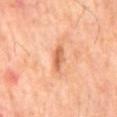No biopsy was performed on this lesion — it was imaged during a full skin examination and was not determined to be concerning.
A male subject aged around 65.
From the mid back.
Cropped from a total-body skin-imaging series; the visible field is about 15 mm.
The tile uses cross-polarized illumination.
About 4 mm across.